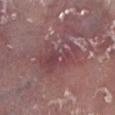biopsy_status: not biopsied; imaged during a skin examination
automated_metrics:
  area_mm2_approx: 15.0
  eccentricity: 0.85
  shape_asymmetry: 0.3
  nevus_likeness_0_100: 0
  lesion_detection_confidence_0_100: 75
patient:
  sex: male
  age_approx: 65
site: left lower leg
lighting: white-light
lesion_size:
  long_diameter_mm_approx: 6.5
image:
  source: total-body photography crop
  field_of_view_mm: 15